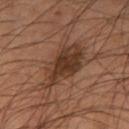Assessment: The lesion was tiled from a total-body skin photograph and was not biopsied. Context: About 6.5 mm across. A male patient aged around 55. A roughly 15 mm field-of-view crop from a total-body skin photograph. The lesion is located on the left thigh. Imaged with cross-polarized lighting.